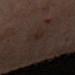  biopsy_status: not biopsied; imaged during a skin examination
  lighting: cross-polarized
  lesion_size:
    long_diameter_mm_approx: 3.0
  patient:
    sex: female
    age_approx: 55
  site: arm
  image:
    source: total-body photography crop
    field_of_view_mm: 15